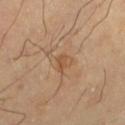This lesion was catalogued during total-body skin photography and was not selected for biopsy.
Captured under cross-polarized illumination.
A male subject about 65 years old.
The lesion is on the left lower leg.
The lesion-visualizer software estimated an average lesion color of about L≈52 a*≈21 b*≈35 (CIELAB), about 7 CIELAB-L* units darker than the surrounding skin, and a normalized lesion–skin contrast near 6.
A 15 mm close-up extracted from a 3D total-body photography capture.